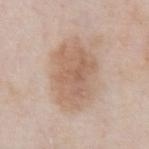Findings:
• workup · no biopsy performed (imaged during a skin exam)
• location · the chest
• subject · male, roughly 55 years of age
• acquisition · ~15 mm crop, total-body skin-cancer survey
• tile lighting · white-light illumination
• lesion diameter · about 7.5 mm
• automated metrics · a lesion area of about 25 mm², an eccentricity of roughly 0.75, and a symmetry-axis asymmetry near 0.2